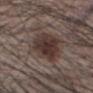Q: Was this lesion biopsied?
A: catalogued during a skin exam; not biopsied
Q: Illumination type?
A: white-light
Q: Lesion size?
A: ≈5 mm
Q: Automated lesion metrics?
A: a lesion area of about 16 mm² and a shape-asymmetry score of about 0.15 (0 = symmetric); border irregularity of about 2 on a 0–10 scale, a within-lesion color-variation index near 3.5/10, and peripheral color asymmetry of about 1; a classifier nevus-likeness of about 85/100 and a detector confidence of about 100 out of 100 that the crop contains a lesion
Q: What kind of image is this?
A: 15 mm crop, total-body photography
Q: Lesion location?
A: the head or neck
Q: Who is the patient?
A: male, approximately 50 years of age